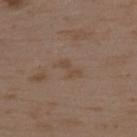Q: Is there a histopathology result?
A: total-body-photography surveillance lesion; no biopsy
Q: Patient demographics?
A: female, in their mid-30s
Q: How was the tile lit?
A: white-light illumination
Q: What is the imaging modality?
A: ~15 mm tile from a whole-body skin photo
Q: Lesion location?
A: the upper back